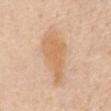<case>
<biopsy_status>not biopsied; imaged during a skin examination</biopsy_status>
<site>abdomen</site>
<automated_metrics>
  <peripheral_color_asymmetry>1.0</peripheral_color_asymmetry>
  <nevus_likeness_0_100>20</nevus_likeness_0_100>
  <lesion_detection_confidence_0_100>100</lesion_detection_confidence_0_100>
</automated_metrics>
<lesion_size>
  <long_diameter_mm_approx>7.0</long_diameter_mm_approx>
</lesion_size>
<image>
  <source>total-body photography crop</source>
  <field_of_view_mm>15</field_of_view_mm>
</image>
<patient>
  <sex>female</sex>
  <age_approx>65</age_approx>
</patient>
</case>